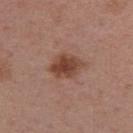| feature | finding |
|---|---|
| notes | total-body-photography surveillance lesion; no biopsy |
| image | 15 mm crop, total-body photography |
| anatomic site | the arm |
| patient | female, about 55 years old |
| lesion size | ~4 mm (longest diameter) |
| tile lighting | white-light |